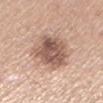No biopsy was performed on this lesion — it was imaged during a full skin examination and was not determined to be concerning. Imaged with white-light lighting. An algorithmic analysis of the crop reported an eccentricity of roughly 0.6 and a shape-asymmetry score of about 0.2 (0 = symmetric). The analysis additionally found radial color variation of about 2. A 15 mm crop from a total-body photograph taken for skin-cancer surveillance. A female subject aged approximately 55. About 5.5 mm across. Located on the left lower leg.